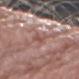Findings:
- biopsy status: catalogued during a skin exam; not biopsied
- automated lesion analysis: an average lesion color of about L≈51 a*≈22 b*≈25 (CIELAB), about 7 CIELAB-L* units darker than the surrounding skin, and a normalized lesion–skin contrast near 5.5
- size: ~2.5 mm (longest diameter)
- lighting: white-light
- patient: male, aged around 80
- acquisition: 15 mm crop, total-body photography
- anatomic site: the right forearm